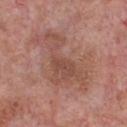The lesion was tiled from a total-body skin photograph and was not biopsied. Longest diameter approximately 8 mm. A male patient, in their 60s. Cropped from a total-body skin-imaging series; the visible field is about 15 mm. On the chest.